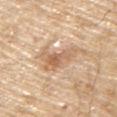Acquisition and patient details: From the upper back. About 5 mm across. A male patient, about 70 years old. Imaged with white-light lighting. A 15 mm close-up tile from a total-body photography series done for melanoma screening.